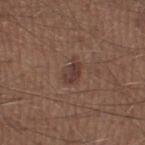notes: catalogued during a skin exam; not biopsied | image source: 15 mm crop, total-body photography | patient: male, in their 30s | location: the left thigh | lighting: white-light.